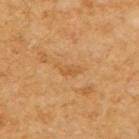Captured during whole-body skin photography for melanoma surveillance; the lesion was not biopsied. About 3 mm across. Located on the right upper arm. A female patient, aged around 55. A close-up tile cropped from a whole-body skin photograph, about 15 mm across.